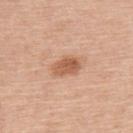Notes:
* workup — no biopsy performed (imaged during a skin exam)
* subject — male, approximately 60 years of age
* body site — the upper back
* image — total-body-photography crop, ~15 mm field of view